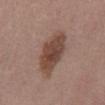Notes:
• biopsy status · no biopsy performed (imaged during a skin exam)
• patient · male, in their 40s
• image source · 15 mm crop, total-body photography
• anatomic site · the abdomen
• automated lesion analysis · a lesion–skin lightness drop of about 12 and a lesion-to-skin contrast of about 9 (normalized; higher = more distinct); border irregularity of about 2 on a 0–10 scale and a peripheral color-asymmetry measure near 1; a classifier nevus-likeness of about 80/100 and lesion-presence confidence of about 100/100
• lesion size · ~6 mm (longest diameter)